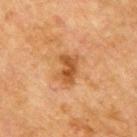Case summary:
• workup · catalogued during a skin exam; not biopsied
• body site · the back
• image source · 15 mm crop, total-body photography
• illumination · cross-polarized
• patient · male, roughly 70 years of age
• lesion diameter · ~3.5 mm (longest diameter)